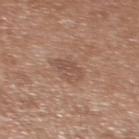Recorded during total-body skin imaging; not selected for excision or biopsy. Longest diameter approximately 3 mm. A female patient approximately 40 years of age. On the upper back. This is a white-light tile. This image is a 15 mm lesion crop taken from a total-body photograph.